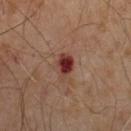The subject is a male aged 63–67. A roughly 15 mm field-of-view crop from a total-body skin photograph. About 3 mm across. The tile uses cross-polarized illumination.